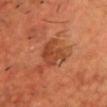Clinical impression: Imaged during a routine full-body skin examination; the lesion was not biopsied and no histopathology is available. Context: The recorded lesion diameter is about 4 mm. Automated image analysis of the tile measured an area of roughly 9.5 mm² and a shape-asymmetry score of about 0.3 (0 = symmetric). The software also gave a border-irregularity rating of about 4/10, a color-variation rating of about 3/10, and peripheral color asymmetry of about 1. The software also gave an automated nevus-likeness rating near 60 out of 100 and a lesion-detection confidence of about 100/100. Located on the chest. A male patient, aged 43 to 47. A roughly 15 mm field-of-view crop from a total-body skin photograph. Imaged with cross-polarized lighting.